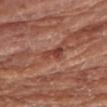Case summary:
* notes — total-body-photography surveillance lesion; no biopsy
* image source — 15 mm crop, total-body photography
* lesion diameter — ≈2.5 mm
* location — the right forearm
* patient — female, aged 73–77
* illumination — white-light